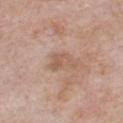{
  "biopsy_status": "not biopsied; imaged during a skin examination",
  "image": {
    "source": "total-body photography crop",
    "field_of_view_mm": 15
  },
  "patient": {
    "sex": "male",
    "age_approx": 60
  },
  "lesion_size": {
    "long_diameter_mm_approx": 3.5
  },
  "site": "chest"
}